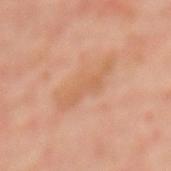Case summary:
• image-analysis metrics: an average lesion color of about L≈63 a*≈22 b*≈36 (CIELAB), roughly 6 lightness units darker than nearby skin, and a normalized border contrast of about 4.5; a detector confidence of about 100 out of 100 that the crop contains a lesion
• body site: the upper back
• lesion size: about 6.5 mm
• image source: ~15 mm tile from a whole-body skin photo
• illumination: cross-polarized illumination
• patient: female, in their 50s